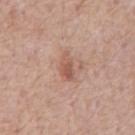workup: total-body-photography surveillance lesion; no biopsy | body site: the chest | lesion size: ~3.5 mm (longest diameter) | subject: male, aged 58 to 62 | TBP lesion metrics: a color-variation rating of about 2.5/10 and peripheral color asymmetry of about 0.5; a detector confidence of about 100 out of 100 that the crop contains a lesion | image source: ~15 mm tile from a whole-body skin photo.